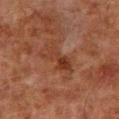This lesion was catalogued during total-body skin photography and was not selected for biopsy.
A lesion tile, about 15 mm wide, cut from a 3D total-body photograph.
The lesion's longest dimension is about 4.5 mm.
The tile uses cross-polarized illumination.
The patient is a male in their 80s.
From the left lower leg.